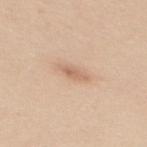acquisition = total-body-photography crop, ~15 mm field of view | subject = female, in their mid- to late 40s | automated lesion analysis = a shape eccentricity near 0.9 and a symmetry-axis asymmetry near 0.25 | tile lighting = white-light illumination | body site = the back.